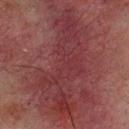The lesion was photographed on a routine skin check and not biopsied; there is no pathology result. A region of skin cropped from a whole-body photographic capture, roughly 15 mm wide. An algorithmic analysis of the crop reported an outline eccentricity of about 0.95 (0 = round, 1 = elongated) and a symmetry-axis asymmetry near 0.5. And it measured a classifier nevus-likeness of about 0/100 and lesion-presence confidence of about 15/100. The tile uses cross-polarized illumination. Measured at roughly 13 mm in maximum diameter. A male subject aged around 65. From the upper back.